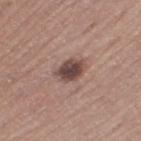Q: Was this lesion biopsied?
A: catalogued during a skin exam; not biopsied
Q: What kind of image is this?
A: ~15 mm crop, total-body skin-cancer survey
Q: What are the patient's age and sex?
A: male, aged 58–62
Q: What did automated image analysis measure?
A: a lesion area of about 6.5 mm², an outline eccentricity of about 0.65 (0 = round, 1 = elongated), and two-axis asymmetry of about 0.2; a border-irregularity index near 2/10, a color-variation rating of about 4.5/10, and radial color variation of about 1
Q: Lesion location?
A: the left thigh
Q: What lighting was used for the tile?
A: white-light illumination